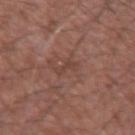Recorded during total-body skin imaging; not selected for excision or biopsy. A roughly 15 mm field-of-view crop from a total-body skin photograph. Located on the left forearm. A male subject roughly 50 years of age.